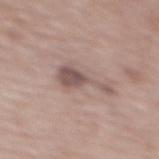tile lighting — white-light illumination | patient — female, about 65 years old | body site — the back | acquisition — 15 mm crop, total-body photography | TBP lesion metrics — an average lesion color of about L≈53 a*≈16 b*≈20 (CIELAB), roughly 11 lightness units darker than nearby skin, and a normalized lesion–skin contrast near 7.5; radial color variation of about 1; a classifier nevus-likeness of about 0/100 and lesion-presence confidence of about 100/100 | size — ~5 mm (longest diameter).